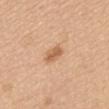| key | value |
|---|---|
| follow-up | total-body-photography surveillance lesion; no biopsy |
| acquisition | total-body-photography crop, ~15 mm field of view |
| patient | female, aged approximately 65 |
| lighting | white-light illumination |
| site | the back |
| size | about 3 mm |